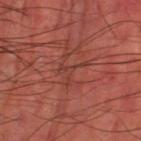Part of a total-body skin-imaging series; this lesion was reviewed on a skin check and was not flagged for biopsy.
A roughly 15 mm field-of-view crop from a total-body skin photograph.
A male subject in their mid- to late 60s.
Located on the leg.
Imaged with cross-polarized lighting.
Approximately 3.5 mm at its widest.
An algorithmic analysis of the crop reported a border-irregularity rating of about 9.5/10. The analysis additionally found a nevus-likeness score of about 0/100 and a lesion-detection confidence of about 50/100.